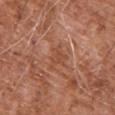Recorded during total-body skin imaging; not selected for excision or biopsy.
A male patient, in their mid- to late 70s.
On the upper back.
A 15 mm crop from a total-body photograph taken for skin-cancer surveillance.